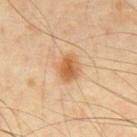subject — male, in their mid-40s | image source — total-body-photography crop, ~15 mm field of view | image-analysis metrics — a mean CIELAB color near L≈57 a*≈21 b*≈39, roughly 11 lightness units darker than nearby skin, and a normalized border contrast of about 8.5; border irregularity of about 1.5 on a 0–10 scale, a within-lesion color-variation index near 4.5/10, and peripheral color asymmetry of about 1.5 | tile lighting — cross-polarized illumination | anatomic site — the front of the torso | lesion size — ~3.5 mm (longest diameter).